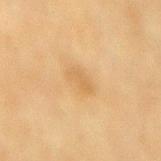Impression: No biopsy was performed on this lesion — it was imaged during a full skin examination and was not determined to be concerning. Context: A female patient, aged 53 to 57. Located on the mid back. The total-body-photography lesion software estimated a footprint of about 4 mm², an eccentricity of roughly 0.85, and a shape-asymmetry score of about 0.4 (0 = symmetric). The analysis additionally found a classifier nevus-likeness of about 0/100 and a detector confidence of about 100 out of 100 that the crop contains a lesion. A region of skin cropped from a whole-body photographic capture, roughly 15 mm wide. Approximately 3 mm at its widest.